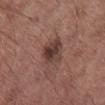Notes:
• follow-up: catalogued during a skin exam; not biopsied
• image-analysis metrics: a lesion area of about 9.5 mm², an outline eccentricity of about 0.65 (0 = round, 1 = elongated), and a shape-asymmetry score of about 0.15 (0 = symmetric); a lesion–skin lightness drop of about 10 and a normalized border contrast of about 8.5; a border-irregularity index near 1.5/10, a within-lesion color-variation index near 6/10, and peripheral color asymmetry of about 2
• patient: male, in their mid- to late 50s
• image: ~15 mm crop, total-body skin-cancer survey
• site: the right lower leg
• lesion size: ≈4 mm